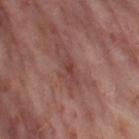Findings:
* notes: no biopsy performed (imaged during a skin exam)
* image source: ~15 mm crop, total-body skin-cancer survey
* illumination: cross-polarized
* anatomic site: the left thigh
* subject: female, about 55 years old
* lesion diameter: about 3 mm